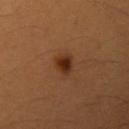Clinical impression: The lesion was photographed on a routine skin check and not biopsied; there is no pathology result. Clinical summary: A female subject aged 38 to 42. The tile uses cross-polarized illumination. Approximately 3 mm at its widest. A 15 mm close-up tile from a total-body photography series done for melanoma screening. The lesion is on the left upper arm.